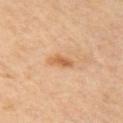Q: Is there a histopathology result?
A: no biopsy performed (imaged during a skin exam)
Q: Patient demographics?
A: male, approximately 45 years of age
Q: Automated lesion metrics?
A: a lesion area of about 4 mm², an outline eccentricity of about 0.85 (0 = round, 1 = elongated), and two-axis asymmetry of about 0.25; an automated nevus-likeness rating near 40 out of 100 and lesion-presence confidence of about 100/100
Q: What kind of image is this?
A: total-body-photography crop, ~15 mm field of view
Q: Illumination type?
A: cross-polarized illumination
Q: Where on the body is the lesion?
A: the right upper arm
Q: What is the lesion's diameter?
A: ≈3 mm